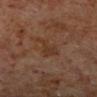Notes:
* biopsy status · imaged on a skin check; not biopsied
* size · ~4 mm (longest diameter)
* location · the left lower leg
* acquisition · total-body-photography crop, ~15 mm field of view
* patient · male, about 60 years old
* tile lighting · cross-polarized